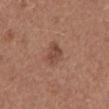Clinical impression:
Recorded during total-body skin imaging; not selected for excision or biopsy.
Image and clinical context:
A male patient in their mid-60s. The lesion is located on the mid back. This image is a 15 mm lesion crop taken from a total-body photograph.